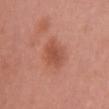workup: total-body-photography surveillance lesion; no biopsy | patient: female, aged around 35 | TBP lesion metrics: a shape-asymmetry score of about 0.2 (0 = symmetric) | acquisition: ~15 mm crop, total-body skin-cancer survey | lighting: white-light illumination | lesion diameter: ~4 mm (longest diameter) | site: the chest.